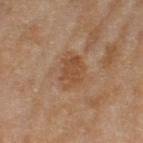Q: Is there a histopathology result?
A: no biopsy performed (imaged during a skin exam)
Q: What is the imaging modality?
A: ~15 mm crop, total-body skin-cancer survey
Q: Where on the body is the lesion?
A: the left thigh
Q: Patient demographics?
A: female, aged 58–62
Q: Lesion size?
A: ~4 mm (longest diameter)
Q: Automated lesion metrics?
A: a footprint of about 13 mm² and an outline eccentricity of about 0.3 (0 = round, 1 = elongated); a border-irregularity rating of about 3/10, a color-variation rating of about 3.5/10, and peripheral color asymmetry of about 1